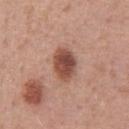Notes:
– workup: imaged on a skin check; not biopsied
– location: the chest
– patient: male, aged 48 to 52
– TBP lesion metrics: an area of roughly 9.5 mm², a shape eccentricity near 0.75, and two-axis asymmetry of about 0.15; a lesion color around L≈49 a*≈23 b*≈27 in CIELAB, roughly 14 lightness units darker than nearby skin, and a normalized border contrast of about 10; a border-irregularity index near 1.5/10 and radial color variation of about 1.5; an automated nevus-likeness rating near 95 out of 100
– image: 15 mm crop, total-body photography
– tile lighting: white-light illumination